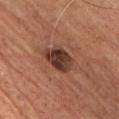The lesion was tiled from a total-body skin photograph and was not biopsied. The lesion-visualizer software estimated a symmetry-axis asymmetry near 0.2. And it measured a border-irregularity rating of about 2/10, a color-variation rating of about 5.5/10, and radial color variation of about 2. It also reported a nevus-likeness score of about 30/100 and a lesion-detection confidence of about 100/100. On the chest. Cropped from a whole-body photographic skin survey; the tile spans about 15 mm. A male subject, roughly 50 years of age.